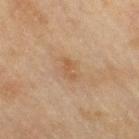This lesion was catalogued during total-body skin photography and was not selected for biopsy. The recorded lesion diameter is about 2.5 mm. A 15 mm crop from a total-body photograph taken for skin-cancer surveillance. The lesion is on the right thigh. The subject is a female about 60 years old. The tile uses cross-polarized illumination.